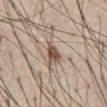No biopsy was performed on this lesion — it was imaged during a full skin examination and was not determined to be concerning. This is a white-light tile. From the chest. About 3.5 mm across. The subject is a male about 60 years old. Cropped from a total-body skin-imaging series; the visible field is about 15 mm.